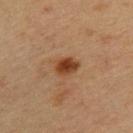Notes:
* workup · no biopsy performed (imaged during a skin exam)
* patient · male, roughly 40 years of age
* anatomic site · the upper back
* image · ~15 mm crop, total-body skin-cancer survey